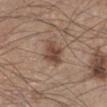* notes — imaged on a skin check; not biopsied